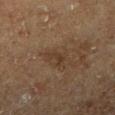This lesion was catalogued during total-body skin photography and was not selected for biopsy. The total-body-photography lesion software estimated a lesion area of about 2.5 mm² and two-axis asymmetry of about 0.55. The analysis additionally found a lesion color around L≈27 a*≈13 b*≈23 in CIELAB, a lesion–skin lightness drop of about 5, and a lesion-to-skin contrast of about 6 (normalized; higher = more distinct). The software also gave border irregularity of about 5.5 on a 0–10 scale, internal color variation of about 0 on a 0–10 scale, and a peripheral color-asymmetry measure near 0. Located on the right lower leg. Approximately 2.5 mm at its widest. The patient is a male approximately 75 years of age. This is a cross-polarized tile. Cropped from a total-body skin-imaging series; the visible field is about 15 mm.